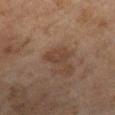| field | value |
|---|---|
| notes | no biopsy performed (imaged during a skin exam) |
| diameter | ≈2.5 mm |
| image | 15 mm crop, total-body photography |
| site | the leg |
| patient | female, aged approximately 60 |
| illumination | cross-polarized illumination |